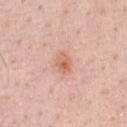Findings:
• follow-up — catalogued during a skin exam; not biopsied
• subject — male, aged 48–52
• image — 15 mm crop, total-body photography
• anatomic site — the chest